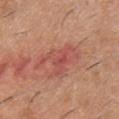biopsy status: no biopsy performed (imaged during a skin exam) | acquisition: total-body-photography crop, ~15 mm field of view | patient: male, approximately 40 years of age | location: the chest.